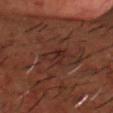The patient is a male aged 48–52.
Longest diameter approximately 3 mm.
A roughly 15 mm field-of-view crop from a total-body skin photograph.
Automated tile analysis of the lesion measured a shape eccentricity near 0.75 and a symmetry-axis asymmetry near 0.4. The analysis additionally found an average lesion color of about L≈20 a*≈17 b*≈18 (CIELAB), a lesion–skin lightness drop of about 4, and a normalized border contrast of about 6. The software also gave a border-irregularity index near 3.5/10.
On the head or neck.